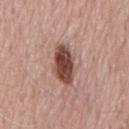  biopsy_status: not biopsied; imaged during a skin examination
  patient:
    sex: male
    age_approx: 75
  site: chest
  image:
    source: total-body photography crop
    field_of_view_mm: 15